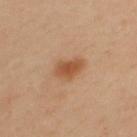<case>
<biopsy_status>not biopsied; imaged during a skin examination</biopsy_status>
<patient>
  <sex>male</sex>
  <age_approx>30</age_approx>
</patient>
<automated_metrics>
  <area_mm2_approx>6.0</area_mm2_approx>
  <shape_asymmetry>0.2</shape_asymmetry>
  <cielab_L>53</cielab_L>
  <cielab_a>23</cielab_a>
  <cielab_b>36</cielab_b>
  <vs_skin_darker_L>11.0</vs_skin_darker_L>
  <vs_skin_contrast_norm>8.0</vs_skin_contrast_norm>
  <border_irregularity_0_10>2.0</border_irregularity_0_10>
  <nevus_likeness_0_100>95</nevus_likeness_0_100>
  <lesion_detection_confidence_0_100>100</lesion_detection_confidence_0_100>
</automated_metrics>
<image>
  <source>total-body photography crop</source>
  <field_of_view_mm>15</field_of_view_mm>
</image>
<site>upper back</site>
<lesion_size>
  <long_diameter_mm_approx>3.5</long_diameter_mm_approx>
</lesion_size>
<lighting>cross-polarized</lighting>
</case>